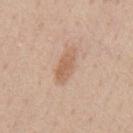follow-up: imaged on a skin check; not biopsied | subject: male, aged 58–62 | location: the back | lesion size: about 4.5 mm | image: total-body-photography crop, ~15 mm field of view | lighting: white-light | image-analysis metrics: an area of roughly 6.5 mm², an outline eccentricity of about 0.9 (0 = round, 1 = elongated), and a shape-asymmetry score of about 0.2 (0 = symmetric); a border-irregularity index near 2.5/10 and a within-lesion color-variation index near 2/10; a detector confidence of about 100 out of 100 that the crop contains a lesion.